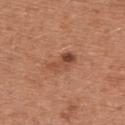No biopsy was performed on this lesion — it was imaged during a full skin examination and was not determined to be concerning.